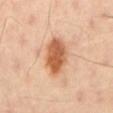No biopsy was performed on this lesion — it was imaged during a full skin examination and was not determined to be concerning. The patient is a male aged 38 to 42. A roughly 15 mm field-of-view crop from a total-body skin photograph. Captured under cross-polarized illumination. The lesion is on the mid back. About 5 mm across.